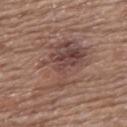Q: Was a biopsy performed?
A: catalogued during a skin exam; not biopsied
Q: What lighting was used for the tile?
A: white-light
Q: Automated lesion metrics?
A: a lesion color around L≈46 a*≈18 b*≈23 in CIELAB and about 8 CIELAB-L* units darker than the surrounding skin; a within-lesion color-variation index near 7.5/10; a classifier nevus-likeness of about 15/100 and a detector confidence of about 100 out of 100 that the crop contains a lesion
Q: How was this image acquired?
A: ~15 mm tile from a whole-body skin photo
Q: Where on the body is the lesion?
A: the upper back
Q: Lesion size?
A: about 8 mm
Q: What are the patient's age and sex?
A: female, in their mid- to late 60s